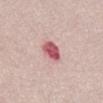This lesion was catalogued during total-body skin photography and was not selected for biopsy.
A male subject approximately 65 years of age.
From the abdomen.
The tile uses white-light illumination.
The lesion-visualizer software estimated a footprint of about 6 mm², a shape eccentricity near 0.6, and a symmetry-axis asymmetry near 0.2.
Cropped from a total-body skin-imaging series; the visible field is about 15 mm.